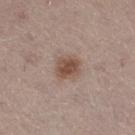Findings:
* location: the right thigh
* image-analysis metrics: a border-irregularity index near 1/10, a within-lesion color-variation index near 3.5/10, and radial color variation of about 1; a nevus-likeness score of about 95/100 and lesion-presence confidence of about 100/100
* diameter: ~3 mm (longest diameter)
* image source: ~15 mm tile from a whole-body skin photo
* patient: male, aged 63–67